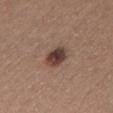follow-up: imaged on a skin check; not biopsied
diameter: ~3.5 mm (longest diameter)
TBP lesion metrics: a border-irregularity index near 2/10, internal color variation of about 4.5 on a 0–10 scale, and a peripheral color-asymmetry measure near 1.5; a classifier nevus-likeness of about 95/100
image: ~15 mm crop, total-body skin-cancer survey
patient: female, about 30 years old
anatomic site: the head or neck
illumination: white-light